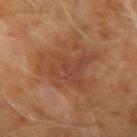{"biopsy_status": "not biopsied; imaged during a skin examination", "image": {"source": "total-body photography crop", "field_of_view_mm": 15}, "patient": {"sex": "male", "age_approx": 70}, "site": "arm"}